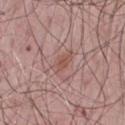Background:
An algorithmic analysis of the crop reported a lesion area of about 4.5 mm², an eccentricity of roughly 0.9, and a symmetry-axis asymmetry near 0.3. The analysis additionally found a mean CIELAB color near L≈52 a*≈22 b*≈25, roughly 7 lightness units darker than nearby skin, and a normalized lesion–skin contrast near 6. This image is a 15 mm lesion crop taken from a total-body photograph. A male subject, in their mid- to late 60s. The lesion's longest dimension is about 3.5 mm. Located on the front of the torso. Imaged with white-light lighting.